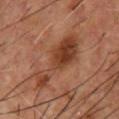| key | value |
|---|---|
| workup | total-body-photography surveillance lesion; no biopsy |
| anatomic site | the chest |
| diameter | ~8.5 mm (longest diameter) |
| patient | male, aged 53–57 |
| imaging modality | ~15 mm crop, total-body skin-cancer survey |
| image-analysis metrics | a lesion area of about 19 mm², an outline eccentricity of about 0.95 (0 = round, 1 = elongated), and a symmetry-axis asymmetry near 0.45; an average lesion color of about L≈40 a*≈23 b*≈31 (CIELAB) and a lesion–skin lightness drop of about 10; a nevus-likeness score of about 0/100 |